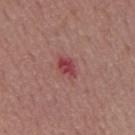Notes:
* workup: total-body-photography surveillance lesion; no biopsy
* body site: the mid back
* acquisition: ~15 mm crop, total-body skin-cancer survey
* patient: male, roughly 70 years of age